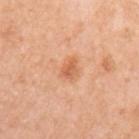Q: What kind of image is this?
A: ~15 mm crop, total-body skin-cancer survey
Q: Where on the body is the lesion?
A: the left upper arm
Q: What lighting was used for the tile?
A: white-light
Q: What is the lesion's diameter?
A: about 2.5 mm
Q: What are the patient's age and sex?
A: female, in their mid- to late 50s
Q: What did automated image analysis measure?
A: an area of roughly 4 mm², an eccentricity of roughly 0.7, and a shape-asymmetry score of about 0.3 (0 = symmetric)